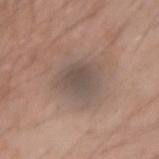workup: imaged on a skin check; not biopsied | site: the right forearm | lesion diameter: ~3.5 mm (longest diameter) | imaging modality: total-body-photography crop, ~15 mm field of view | tile lighting: white-light | patient: male, about 30 years old | automated metrics: a shape eccentricity near 0.45 and a symmetry-axis asymmetry near 0.2; an average lesion color of about L≈49 a*≈12 b*≈21 (CIELAB), a lesion–skin lightness drop of about 8, and a normalized border contrast of about 6.5; a nevus-likeness score of about 0/100 and lesion-presence confidence of about 85/100.